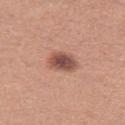Case summary:
• body site — the leg
• tile lighting — white-light
• TBP lesion metrics — a mean CIELAB color near L≈51 a*≈23 b*≈26, roughly 14 lightness units darker than nearby skin, and a lesion-to-skin contrast of about 9.5 (normalized; higher = more distinct); a within-lesion color-variation index near 4.5/10 and a peripheral color-asymmetry measure near 1; lesion-presence confidence of about 100/100
• lesion size — ~3.5 mm (longest diameter)
• patient — female, roughly 30 years of age
• image — ~15 mm crop, total-body skin-cancer survey